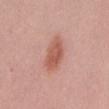This lesion was catalogued during total-body skin photography and was not selected for biopsy. The patient is a female about 40 years old. The total-body-photography lesion software estimated a footprint of about 9.5 mm², an outline eccentricity of about 0.85 (0 = round, 1 = elongated), and two-axis asymmetry of about 0.2. The software also gave about 11 CIELAB-L* units darker than the surrounding skin and a normalized border contrast of about 7.5. And it measured a color-variation rating of about 3/10 and a peripheral color-asymmetry measure near 1. And it measured lesion-presence confidence of about 100/100. Captured under white-light illumination. A region of skin cropped from a whole-body photographic capture, roughly 15 mm wide. Located on the mid back. Measured at roughly 5 mm in maximum diameter.